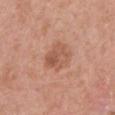The lesion was tiled from a total-body skin photograph and was not biopsied. A male subject aged around 70. A 15 mm crop from a total-body photograph taken for skin-cancer surveillance. From the left upper arm. Captured under white-light illumination. About 4.5 mm across.